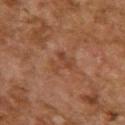<lesion>
<biopsy_status>not biopsied; imaged during a skin examination</biopsy_status>
<image>
  <source>total-body photography crop</source>
  <field_of_view_mm>15</field_of_view_mm>
</image>
<patient>
  <sex>female</sex>
  <age_approx>60</age_approx>
</patient>
<automated_metrics>
  <eccentricity>0.5</eccentricity>
  <shape_asymmetry>0.55</shape_asymmetry>
  <cielab_L>37</cielab_L>
  <cielab_a>21</cielab_a>
  <cielab_b>28</cielab_b>
  <vs_skin_darker_L>6.0</vs_skin_darker_L>
  <vs_skin_contrast_norm>5.5</vs_skin_contrast_norm>
  <color_variation_0_10>1.5</color_variation_0_10>
  <peripheral_color_asymmetry>0.5</peripheral_color_asymmetry>
  <nevus_likeness_0_100>0</nevus_likeness_0_100>
  <lesion_detection_confidence_0_100>100</lesion_detection_confidence_0_100>
</automated_metrics>
<lesion_size>
  <long_diameter_mm_approx>3.0</long_diameter_mm_approx>
</lesion_size>
<lighting>cross-polarized</lighting>
<site>upper back</site>
</lesion>